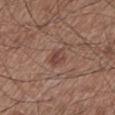This lesion was catalogued during total-body skin photography and was not selected for biopsy. The tile uses white-light illumination. A 15 mm crop from a total-body photograph taken for skin-cancer surveillance. A male subject aged 58 to 62. Approximately 2.5 mm at its widest. Automated tile analysis of the lesion measured an area of roughly 3.5 mm², an eccentricity of roughly 0.75, and a shape-asymmetry score of about 0.25 (0 = symmetric). From the leg.